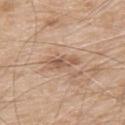{"biopsy_status": "not biopsied; imaged during a skin examination", "patient": {"sex": "male", "age_approx": 80}, "site": "upper back", "automated_metrics": {"cielab_L": 56, "cielab_a": 19, "cielab_b": 30, "vs_skin_darker_L": 10.0}, "lesion_size": {"long_diameter_mm_approx": 3.5}, "image": {"source": "total-body photography crop", "field_of_view_mm": 15}}